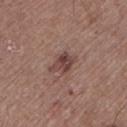body site — the left lower leg
image — total-body-photography crop, ~15 mm field of view
subject — male, in their mid- to late 60s